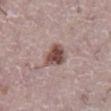workup: no biopsy performed (imaged during a skin exam); lesion size: ≈3 mm; image source: total-body-photography crop, ~15 mm field of view; illumination: white-light; subject: male, in their mid- to late 70s; anatomic site: the abdomen.